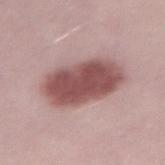<case>
<image>
  <source>total-body photography crop</source>
  <field_of_view_mm>15</field_of_view_mm>
</image>
<automated_metrics>
  <area_mm2_approx>26.0</area_mm2_approx>
  <eccentricity>0.85</eccentricity>
  <shape_asymmetry>0.1</shape_asymmetry>
  <cielab_L>51</cielab_L>
  <cielab_a>23</cielab_a>
  <cielab_b>20</cielab_b>
  <nevus_likeness_0_100>90</nevus_likeness_0_100>
  <lesion_detection_confidence_0_100>100</lesion_detection_confidence_0_100>
</automated_metrics>
<lighting>white-light</lighting>
<lesion_size>
  <long_diameter_mm_approx>7.5</long_diameter_mm_approx>
</lesion_size>
<patient>
  <sex>male</sex>
  <age_approx>30</age_approx>
</patient>
</case>